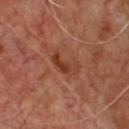Imaged during a routine full-body skin examination; the lesion was not biopsied and no histopathology is available. A male subject, approximately 65 years of age. A region of skin cropped from a whole-body photographic capture, roughly 15 mm wide. Longest diameter approximately 3.5 mm.